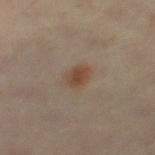Clinical impression:
The lesion was tiled from a total-body skin photograph and was not biopsied.
Image and clinical context:
About 2.5 mm across. A male subject, aged approximately 60. The lesion is on the right thigh. A 15 mm close-up tile from a total-body photography series done for melanoma screening. Automated tile analysis of the lesion measured a border-irregularity rating of about 2/10 and a color-variation rating of about 2.5/10.